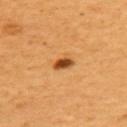This lesion was catalogued during total-body skin photography and was not selected for biopsy.
The lesion is located on the upper back.
A lesion tile, about 15 mm wide, cut from a 3D total-body photograph.
A female patient, aged 53 to 57.